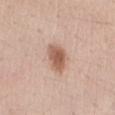| feature | finding |
|---|---|
| biopsy status | total-body-photography surveillance lesion; no biopsy |
| acquisition | ~15 mm tile from a whole-body skin photo |
| subject | female, in their mid-30s |
| lesion diameter | ~4 mm (longest diameter) |
| site | the abdomen |
| lighting | white-light illumination |